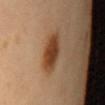Q: Is there a histopathology result?
A: catalogued during a skin exam; not biopsied
Q: Patient demographics?
A: female, aged 63 to 67
Q: What kind of image is this?
A: ~15 mm tile from a whole-body skin photo
Q: How was the tile lit?
A: cross-polarized illumination
Q: What is the lesion's diameter?
A: ≈4.5 mm
Q: Lesion location?
A: the back